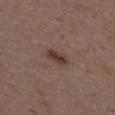Assessment:
This lesion was catalogued during total-body skin photography and was not selected for biopsy.
Image and clinical context:
A 15 mm crop from a total-body photograph taken for skin-cancer surveillance. A male patient in their 50s. The lesion is located on the upper back. An algorithmic analysis of the crop reported a footprint of about 4.5 mm², a shape eccentricity near 0.85, and a symmetry-axis asymmetry near 0.35. The analysis additionally found a mean CIELAB color near L≈37 a*≈17 b*≈24 and a normalized lesion–skin contrast near 8.5. Longest diameter approximately 3 mm.